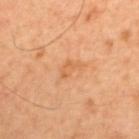| field | value |
|---|---|
| biopsy status | no biopsy performed (imaged during a skin exam) |
| size | ≈3 mm |
| acquisition | total-body-photography crop, ~15 mm field of view |
| location | the upper back |
| subject | male, aged 58 to 62 |
| lighting | cross-polarized illumination |